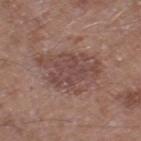{"biopsy_status": "not biopsied; imaged during a skin examination", "patient": {"sex": "male", "age_approx": 60}, "site": "right thigh", "image": {"source": "total-body photography crop", "field_of_view_mm": 15}, "lighting": "white-light"}